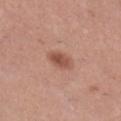automated metrics: a border-irregularity rating of about 1.5/10 and a peripheral color-asymmetry measure near 1.5 | illumination: white-light illumination | patient: female, in their 30s | acquisition: total-body-photography crop, ~15 mm field of view | body site: the right lower leg.